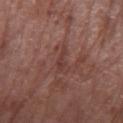notes: imaged on a skin check; not biopsied | anatomic site: the left forearm | illumination: white-light illumination | diameter: ≈2.5 mm | patient: female, in their 70s | image source: 15 mm crop, total-body photography.